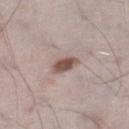Part of a total-body skin-imaging series; this lesion was reviewed on a skin check and was not flagged for biopsy.
Located on the right lower leg.
A roughly 15 mm field-of-view crop from a total-body skin photograph.
A male subject, aged around 70.